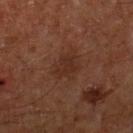• illumination · cross-polarized
• size · ≈2.5 mm
• acquisition · ~15 mm tile from a whole-body skin photo
• TBP lesion metrics · an area of roughly 4.5 mm², a shape eccentricity near 0.45, and two-axis asymmetry of about 0.4; an average lesion color of about L≈26 a*≈19 b*≈24 (CIELAB), roughly 5 lightness units darker than nearby skin, and a lesion-to-skin contrast of about 5.5 (normalized; higher = more distinct); a classifier nevus-likeness of about 5/100 and lesion-presence confidence of about 100/100
• location · the right lower leg
• patient · male, aged 58–62